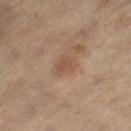Part of a total-body skin-imaging series; this lesion was reviewed on a skin check and was not flagged for biopsy. The lesion-visualizer software estimated a normalized lesion–skin contrast near 5.5. It also reported lesion-presence confidence of about 100/100. On the left thigh. Imaged with cross-polarized lighting. A 15 mm close-up tile from a total-body photography series done for melanoma screening. Measured at roughly 2.5 mm in maximum diameter. The subject is a male aged 58–62.